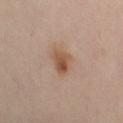Notes:
– workup — imaged on a skin check; not biopsied
– TBP lesion metrics — a footprint of about 5 mm², an eccentricity of roughly 0.7, and a shape-asymmetry score of about 0.3 (0 = symmetric); a lesion color around L≈52 a*≈19 b*≈31 in CIELAB, a lesion–skin lightness drop of about 10, and a normalized border contrast of about 8.5; a border-irregularity rating of about 2.5/10 and a peripheral color-asymmetry measure near 1.5
– site — the right leg
– lesion size — ≈3 mm
– subject — female, about 40 years old
– lighting — cross-polarized illumination
– image source — ~15 mm tile from a whole-body skin photo